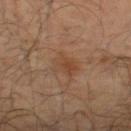Q: Is there a histopathology result?
A: catalogued during a skin exam; not biopsied
Q: What did automated image analysis measure?
A: a lesion area of about 6 mm²
Q: Lesion location?
A: the right lower leg
Q: What is the imaging modality?
A: ~15 mm crop, total-body skin-cancer survey
Q: What lighting was used for the tile?
A: cross-polarized illumination
Q: How large is the lesion?
A: about 3 mm
Q: Patient demographics?
A: male, in their mid- to late 60s